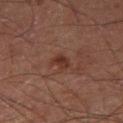* follow-up: total-body-photography surveillance lesion; no biopsy
* location: the right thigh
* image source: 15 mm crop, total-body photography
* image-analysis metrics: a lesion area of about 3 mm² and two-axis asymmetry of about 0.3; an average lesion color of about L≈30 a*≈21 b*≈24 (CIELAB)
* subject: male, aged 58 to 62